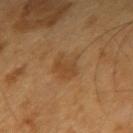workup: no biopsy performed (imaged during a skin exam) | patient: male, roughly 60 years of age | site: the arm | size: about 3.5 mm | image-analysis metrics: a mean CIELAB color near L≈44 a*≈19 b*≈36 and roughly 6 lightness units darker than nearby skin; a lesion-detection confidence of about 100/100 | image: 15 mm crop, total-body photography | tile lighting: cross-polarized.